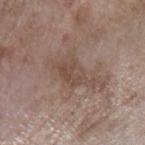| feature | finding |
|---|---|
| biopsy status | imaged on a skin check; not biopsied |
| imaging modality | 15 mm crop, total-body photography |
| size | ≈5 mm |
| site | the left forearm |
| patient | female, in their mid- to late 70s |